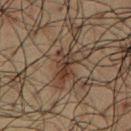The lesion was photographed on a routine skin check and not biopsied; there is no pathology result.
A 15 mm close-up extracted from a 3D total-body photography capture.
The lesion's longest dimension is about 4 mm.
A male subject aged around 60.
On the chest.